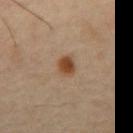<record>
<biopsy_status>not biopsied; imaged during a skin examination</biopsy_status>
<site>mid back</site>
<image>
  <source>total-body photography crop</source>
  <field_of_view_mm>15</field_of_view_mm>
</image>
<lesion_size>
  <long_diameter_mm_approx>2.5</long_diameter_mm_approx>
</lesion_size>
<automated_metrics>
  <border_irregularity_0_10>2.0</border_irregularity_0_10>
  <color_variation_0_10>3.0</color_variation_0_10>
  <peripheral_color_asymmetry>1.0</peripheral_color_asymmetry>
  <nevus_likeness_0_100>100</nevus_likeness_0_100>
  <lesion_detection_confidence_0_100>100</lesion_detection_confidence_0_100>
</automated_metrics>
<patient>
  <sex>male</sex>
  <age_approx>45</age_approx>
</patient>
</record>